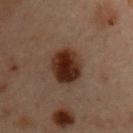<tbp_lesion>
  <biopsy_status>not biopsied; imaged during a skin examination</biopsy_status>
  <lighting>cross-polarized</lighting>
  <lesion_size>
    <long_diameter_mm_approx>4.0</long_diameter_mm_approx>
  </lesion_size>
  <patient>
    <sex>male</sex>
    <age_approx>55</age_approx>
  </patient>
  <site>chest</site>
  <image>
    <source>total-body photography crop</source>
    <field_of_view_mm>15</field_of_view_mm>
  </image>
  <automated_metrics>
    <eccentricity>0.45</eccentricity>
    <shape_asymmetry>0.1</shape_asymmetry>
    <cielab_L>22</cielab_L>
    <cielab_a>16</cielab_a>
    <cielab_b>21</cielab_b>
    <vs_skin_contrast_norm>13.5</vs_skin_contrast_norm>
  </automated_metrics>
</tbp_lesion>